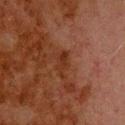biopsy status: imaged on a skin check; not biopsied
size: ≈3 mm
TBP lesion metrics: an area of roughly 4 mm² and two-axis asymmetry of about 0.45; an automated nevus-likeness rating near 0 out of 100 and a lesion-detection confidence of about 100/100
location: the upper back
subject: male, aged around 80
image source: ~15 mm tile from a whole-body skin photo